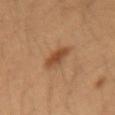Captured during whole-body skin photography for melanoma surveillance; the lesion was not biopsied. This image is a 15 mm lesion crop taken from a total-body photograph. A female subject, aged 33–37. The tile uses cross-polarized illumination. The lesion is on the right forearm.